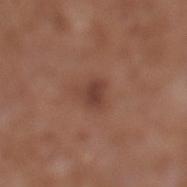follow-up = no biopsy performed (imaged during a skin exam)
location = the left lower leg
lighting = white-light illumination
diameter = ~2.5 mm (longest diameter)
patient = male, about 75 years old
automated metrics = an eccentricity of roughly 0.5 and a symmetry-axis asymmetry near 0.4; border irregularity of about 3.5 on a 0–10 scale, a within-lesion color-variation index near 1.5/10, and peripheral color asymmetry of about 0.5; lesion-presence confidence of about 100/100
image = 15 mm crop, total-body photography